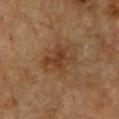A lesion tile, about 15 mm wide, cut from a 3D total-body photograph. A female subject, aged around 55. Longest diameter approximately 3.5 mm. The tile uses cross-polarized illumination. From the chest.